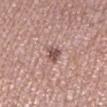| field | value |
|---|---|
| workup | total-body-photography surveillance lesion; no biopsy |
| acquisition | ~15 mm crop, total-body skin-cancer survey |
| TBP lesion metrics | a color-variation rating of about 1.5/10 |
| body site | the left lower leg |
| diameter | ≈2.5 mm |
| patient | female, roughly 60 years of age |
| tile lighting | white-light |